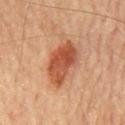Imaged during a routine full-body skin examination; the lesion was not biopsied and no histopathology is available. Located on the chest. This image is a 15 mm lesion crop taken from a total-body photograph. The patient is a male aged 63–67.